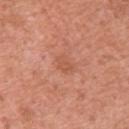Q: Is there a histopathology result?
A: total-body-photography surveillance lesion; no biopsy
Q: Illumination type?
A: white-light
Q: What kind of image is this?
A: ~15 mm tile from a whole-body skin photo
Q: What are the patient's age and sex?
A: female, in their mid- to late 30s
Q: What is the anatomic site?
A: the back